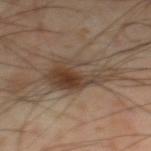Q: Is there a histopathology result?
A: catalogued during a skin exam; not biopsied
Q: What lighting was used for the tile?
A: cross-polarized illumination
Q: Lesion size?
A: ≈7 mm
Q: Where on the body is the lesion?
A: the left lower leg
Q: Who is the patient?
A: male, aged 53 to 57
Q: What kind of image is this?
A: total-body-photography crop, ~15 mm field of view